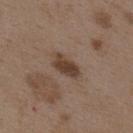This lesion was catalogued during total-body skin photography and was not selected for biopsy. This is a white-light tile. An algorithmic analysis of the crop reported a mean CIELAB color near L≈40 a*≈16 b*≈25, roughly 11 lightness units darker than nearby skin, and a normalized border contrast of about 9.5. It also reported a border-irregularity rating of about 2/10, a within-lesion color-variation index near 3/10, and radial color variation of about 1. The lesion is on the upper back. A 15 mm close-up extracted from a 3D total-body photography capture. Measured at roughly 3.5 mm in maximum diameter. A female patient in their mid-30s.